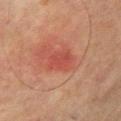{"biopsy_status": "not biopsied; imaged during a skin examination", "site": "arm", "image": {"source": "total-body photography crop", "field_of_view_mm": 15}, "patient": {"sex": "male", "age_approx": 75}}